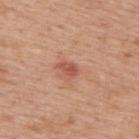Impression: This lesion was catalogued during total-body skin photography and was not selected for biopsy. Image and clinical context: The recorded lesion diameter is about 2.5 mm. This is a white-light tile. A male subject, aged 73–77. Located on the back. A 15 mm close-up extracted from a 3D total-body photography capture.